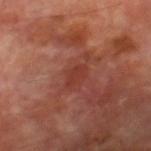Q: Was a biopsy performed?
A: catalogued during a skin exam; not biopsied
Q: What are the patient's age and sex?
A: male, roughly 70 years of age
Q: Where on the body is the lesion?
A: the right lower leg
Q: How was this image acquired?
A: ~15 mm tile from a whole-body skin photo
Q: What is the lesion's diameter?
A: ~2.5 mm (longest diameter)
Q: How was the tile lit?
A: cross-polarized